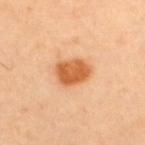follow-up — no biopsy performed (imaged during a skin exam)
image source — ~15 mm tile from a whole-body skin photo
body site — the back
patient — male, roughly 45 years of age